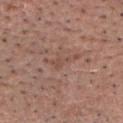<record>
<biopsy_status>not biopsied; imaged during a skin examination</biopsy_status>
<image>
  <source>total-body photography crop</source>
  <field_of_view_mm>15</field_of_view_mm>
</image>
<patient>
  <sex>male</sex>
  <age_approx>65</age_approx>
</patient>
<lesion_size>
  <long_diameter_mm_approx>4.0</long_diameter_mm_approx>
</lesion_size>
<lighting>white-light</lighting>
<site>head or neck</site>
</record>